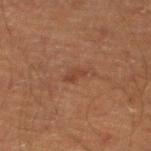Q: Was this lesion biopsied?
A: total-body-photography surveillance lesion; no biopsy
Q: Patient demographics?
A: male, aged approximately 45
Q: Where on the body is the lesion?
A: the leg
Q: What kind of image is this?
A: 15 mm crop, total-body photography
Q: Automated lesion metrics?
A: internal color variation of about 0 on a 0–10 scale and radial color variation of about 0
Q: How was the tile lit?
A: cross-polarized illumination